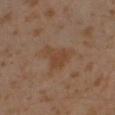notes: no biopsy performed (imaged during a skin exam)
imaging modality: 15 mm crop, total-body photography
patient: male, roughly 30 years of age
anatomic site: the left lower leg